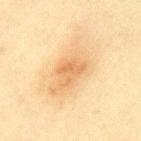Q: Is there a histopathology result?
A: total-body-photography surveillance lesion; no biopsy
Q: How was the tile lit?
A: cross-polarized illumination
Q: What is the imaging modality?
A: total-body-photography crop, ~15 mm field of view
Q: Who is the patient?
A: female, approximately 45 years of age
Q: What is the anatomic site?
A: the mid back
Q: Lesion size?
A: ~4 mm (longest diameter)